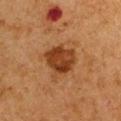Notes:
– notes — imaged on a skin check; not biopsied
– site — the right upper arm
– acquisition — 15 mm crop, total-body photography
– lighting — cross-polarized illumination
– subject — male, in their mid-60s